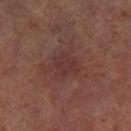Imaged during a routine full-body skin examination; the lesion was not biopsied and no histopathology is available. The recorded lesion diameter is about 2.5 mm. The lesion is on the left lower leg. The lesion-visualizer software estimated a border-irregularity rating of about 3/10, a color-variation rating of about 2/10, and radial color variation of about 0.5. A 15 mm close-up extracted from a 3D total-body photography capture. Captured under cross-polarized illumination. A male subject, aged around 65.